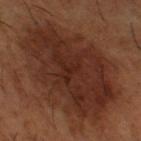The lesion was tiled from a total-body skin photograph and was not biopsied. Located on the leg. A 15 mm close-up extracted from a 3D total-body photography capture. Automated image analysis of the tile measured a classifier nevus-likeness of about 0/100 and lesion-presence confidence of about 100/100. A male patient, roughly 65 years of age.